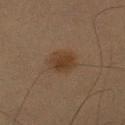Acquisition and patient details: Located on the right upper arm. Cropped from a total-body skin-imaging series; the visible field is about 15 mm. A male subject, aged approximately 65.